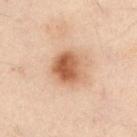workup — imaged on a skin check; not biopsied
automated lesion analysis — an area of roughly 11 mm² and two-axis asymmetry of about 0.2; an average lesion color of about L≈49 a*≈19 b*≈30 (CIELAB), about 13 CIELAB-L* units darker than the surrounding skin, and a lesion-to-skin contrast of about 9.5 (normalized; higher = more distinct)
patient — female, roughly 55 years of age
lesion diameter — ≈4 mm
image — ~15 mm crop, total-body skin-cancer survey
lighting — cross-polarized illumination
body site — the abdomen